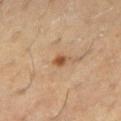Captured during whole-body skin photography for melanoma surveillance; the lesion was not biopsied. Longest diameter approximately 2 mm. Imaged with cross-polarized lighting. From the right thigh. A lesion tile, about 15 mm wide, cut from a 3D total-body photograph. A male patient about 60 years old.